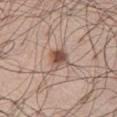The lesion was photographed on a routine skin check and not biopsied; there is no pathology result.
A region of skin cropped from a whole-body photographic capture, roughly 15 mm wide.
The subject is a male about 70 years old.
On the front of the torso.
Imaged with white-light lighting.